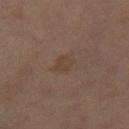The lesion was tiled from a total-body skin photograph and was not biopsied.
The subject is a female aged 58 to 62.
Cropped from a total-body skin-imaging series; the visible field is about 15 mm.
From the right thigh.